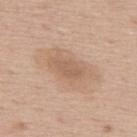workup — no biopsy performed (imaged during a skin exam)
location — the upper back
acquisition — ~15 mm tile from a whole-body skin photo
patient — male, about 60 years old
illumination — white-light illumination